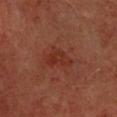biopsy_status: not biopsied; imaged during a skin examination
patient:
  sex: male
  age_approx: 60
lighting: cross-polarized
lesion_size:
  long_diameter_mm_approx: 3.5
site: upper back
image:
  source: total-body photography crop
  field_of_view_mm: 15
automated_metrics:
  nevus_likeness_0_100: 0
  lesion_detection_confidence_0_100: 100